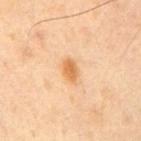Case summary:
* follow-up · total-body-photography surveillance lesion; no biopsy
* anatomic site · the right upper arm
* subject · male, about 40 years old
* diameter · ≈3 mm
* illumination · cross-polarized illumination
* image-analysis metrics · a footprint of about 4.5 mm²
* image source · ~15 mm tile from a whole-body skin photo